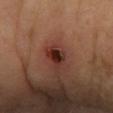follow-up — total-body-photography surveillance lesion; no biopsy
patient — female, roughly 70 years of age
imaging modality — total-body-photography crop, ~15 mm field of view
lighting — cross-polarized illumination
lesion size — ~2.5 mm (longest diameter)
location — the arm